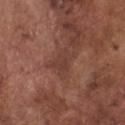A male subject, aged 73 to 77.
This is a white-light tile.
The lesion is on the chest.
A region of skin cropped from a whole-body photographic capture, roughly 15 mm wide.
The lesion's longest dimension is about 2.5 mm.